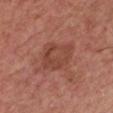Assessment:
The lesion was tiled from a total-body skin photograph and was not biopsied.
Context:
The total-body-photography lesion software estimated border irregularity of about 2 on a 0–10 scale, a within-lesion color-variation index near 4/10, and radial color variation of about 1.5. The patient is a male approximately 60 years of age. A 15 mm close-up tile from a total-body photography series done for melanoma screening. The tile uses white-light illumination. The lesion's longest dimension is about 4.5 mm. On the front of the torso.